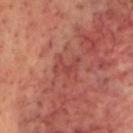Assessment: The lesion was tiled from a total-body skin photograph and was not biopsied. Acquisition and patient details: A lesion tile, about 15 mm wide, cut from a 3D total-body photograph. The lesion is located on the head or neck. Measured at roughly 3 mm in maximum diameter. The total-body-photography lesion software estimated a mean CIELAB color near L≈45 a*≈30 b*≈26 and about 6 CIELAB-L* units darker than the surrounding skin. And it measured a classifier nevus-likeness of about 0/100 and a lesion-detection confidence of about 90/100. A male subject roughly 70 years of age. Captured under cross-polarized illumination.